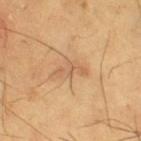Context:
A 15 mm close-up extracted from a 3D total-body photography capture. Imaged with cross-polarized lighting. A male patient, in their mid-60s. On the right thigh. The lesion's longest dimension is about 3.5 mm. The lesion-visualizer software estimated a lesion area of about 4 mm² and a shape-asymmetry score of about 0.45 (0 = symmetric). The software also gave a lesion–skin lightness drop of about 8 and a lesion-to-skin contrast of about 5 (normalized; higher = more distinct). And it measured border irregularity of about 6 on a 0–10 scale, a color-variation rating of about 0.5/10, and a peripheral color-asymmetry measure near 0.